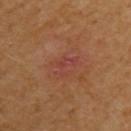Impression: Part of a total-body skin-imaging series; this lesion was reviewed on a skin check and was not flagged for biopsy. Acquisition and patient details: The patient is a female approximately 40 years of age. From the left upper arm. This image is a 15 mm lesion crop taken from a total-body photograph.